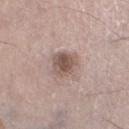The lesion was photographed on a routine skin check and not biopsied; there is no pathology result. A male patient aged around 60. Automated tile analysis of the lesion measured a border-irregularity rating of about 2.5/10, a within-lesion color-variation index near 4.5/10, and a peripheral color-asymmetry measure near 1. It also reported an automated nevus-likeness rating near 65 out of 100. A roughly 15 mm field-of-view crop from a total-body skin photograph. Located on the left lower leg.